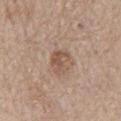workup: total-body-photography surveillance lesion; no biopsy
patient: male, approximately 80 years of age
TBP lesion metrics: a shape eccentricity near 0.8 and a symmetry-axis asymmetry near 0.3; an average lesion color of about L≈53 a*≈18 b*≈28 (CIELAB), about 9 CIELAB-L* units darker than the surrounding skin, and a normalized border contrast of about 7; a border-irregularity index near 3.5/10 and a color-variation rating of about 4/10; a nevus-likeness score of about 0/100 and lesion-presence confidence of about 100/100
size: ~3.5 mm (longest diameter)
image: ~15 mm crop, total-body skin-cancer survey
anatomic site: the mid back
illumination: white-light illumination